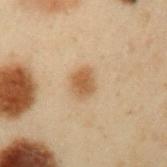Impression: No biopsy was performed on this lesion — it was imaged during a full skin examination and was not determined to be concerning. Acquisition and patient details: On the left upper arm. About 3 mm across. The lesion-visualizer software estimated a footprint of about 5.5 mm² and an outline eccentricity of about 0.65 (0 = round, 1 = elongated). The analysis additionally found a lesion color around L≈46 a*≈16 b*≈31 in CIELAB, about 10 CIELAB-L* units darker than the surrounding skin, and a lesion-to-skin contrast of about 8 (normalized; higher = more distinct). And it measured a nevus-likeness score of about 100/100 and lesion-presence confidence of about 100/100. The patient is a male roughly 55 years of age. This is a cross-polarized tile. A 15 mm close-up extracted from a 3D total-body photography capture.